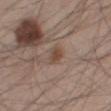Imaged during a routine full-body skin examination; the lesion was not biopsied and no histopathology is available. The total-body-photography lesion software estimated a lesion–skin lightness drop of about 8 and a normalized border contrast of about 7. The analysis additionally found a border-irregularity index near 1.5/10, internal color variation of about 2.5 on a 0–10 scale, and radial color variation of about 1. The tile uses white-light illumination. Longest diameter approximately 2.5 mm. Cropped from a whole-body photographic skin survey; the tile spans about 15 mm. The subject is a male aged 43 to 47. The lesion is located on the leg.